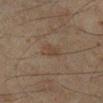No biopsy was performed on this lesion — it was imaged during a full skin examination and was not determined to be concerning.
A male patient, aged around 45.
The lesion's longest dimension is about 2.5 mm.
Captured under cross-polarized illumination.
Located on the leg.
An algorithmic analysis of the crop reported a normalized lesion–skin contrast near 5. The software also gave a within-lesion color-variation index near 1.5/10. It also reported an automated nevus-likeness rating near 5 out of 100 and a detector confidence of about 100 out of 100 that the crop contains a lesion.
A 15 mm crop from a total-body photograph taken for skin-cancer surveillance.